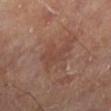notes: total-body-photography surveillance lesion; no biopsy
subject: male, aged around 70
illumination: cross-polarized illumination
size: ~4 mm (longest diameter)
image source: ~15 mm tile from a whole-body skin photo
anatomic site: the left lower leg